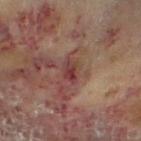Captured during whole-body skin photography for melanoma surveillance; the lesion was not biopsied. The recorded lesion diameter is about 3 mm. The lesion is on the left thigh. A region of skin cropped from a whole-body photographic capture, roughly 15 mm wide. A female subject aged 58 to 62. The tile uses cross-polarized illumination. Automated tile analysis of the lesion measured a lesion color around L≈35 a*≈22 b*≈20 in CIELAB and roughly 9 lightness units darker than nearby skin. And it measured border irregularity of about 3 on a 0–10 scale and internal color variation of about 8 on a 0–10 scale.